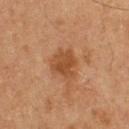Imaged during a routine full-body skin examination; the lesion was not biopsied and no histopathology is available.
About 4 mm across.
A 15 mm close-up tile from a total-body photography series done for melanoma screening.
A male patient, aged around 60.
Imaged with cross-polarized lighting.
An algorithmic analysis of the crop reported a nevus-likeness score of about 15/100 and a lesion-detection confidence of about 100/100.